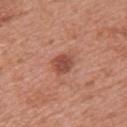Clinical impression: This lesion was catalogued during total-body skin photography and was not selected for biopsy. Background: This is a white-light tile. On the upper back. The total-body-photography lesion software estimated a lesion area of about 5.5 mm², a shape eccentricity near 0.6, and two-axis asymmetry of about 0.2. The analysis additionally found a mean CIELAB color near L≈49 a*≈27 b*≈29 and roughly 11 lightness units darker than nearby skin. The software also gave a border-irregularity rating of about 2/10, internal color variation of about 3 on a 0–10 scale, and peripheral color asymmetry of about 1. And it measured an automated nevus-likeness rating near 75 out of 100. This image is a 15 mm lesion crop taken from a total-body photograph. The lesion's longest dimension is about 3 mm. A female patient aged 38 to 42.